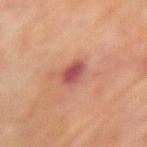biopsy_status: not biopsied; imaged during a skin examination
lesion_size:
  long_diameter_mm_approx: 3.0
image:
  source: total-body photography crop
  field_of_view_mm: 15
site: left upper arm
automated_metrics:
  color_variation_0_10: 3.0
  nevus_likeness_0_100: 5
patient:
  sex: female
  age_approx: 80
lighting: cross-polarized